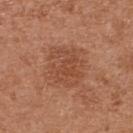Recorded during total-body skin imaging; not selected for excision or biopsy. Captured under white-light illumination. The lesion-visualizer software estimated a symmetry-axis asymmetry near 0.2. And it measured an automated nevus-likeness rating near 55 out of 100. This image is a 15 mm lesion crop taken from a total-body photograph. The patient is a female about 40 years old. The lesion is located on the upper back.